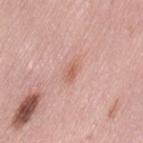Background: The patient is a female roughly 50 years of age. Automated tile analysis of the lesion measured a color-variation rating of about 1.5/10 and radial color variation of about 0.5. It also reported an automated nevus-likeness rating near 0 out of 100 and lesion-presence confidence of about 100/100. The lesion's longest dimension is about 3 mm. The lesion is on the left thigh. A region of skin cropped from a whole-body photographic capture, roughly 15 mm wide.